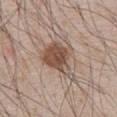| feature | finding |
|---|---|
| biopsy status | catalogued during a skin exam; not biopsied |
| image | ~15 mm crop, total-body skin-cancer survey |
| body site | the chest |
| lesion diameter | ≈5.5 mm |
| image-analysis metrics | a footprint of about 15 mm² and an outline eccentricity of about 0.65 (0 = round, 1 = elongated); a lesion color around L≈51 a*≈17 b*≈26 in CIELAB, a lesion–skin lightness drop of about 12, and a normalized lesion–skin contrast near 9; border irregularity of about 4.5 on a 0–10 scale and peripheral color asymmetry of about 1.5; a nevus-likeness score of about 95/100 and a lesion-detection confidence of about 100/100 |
| subject | male, aged approximately 65 |
| lighting | white-light illumination |